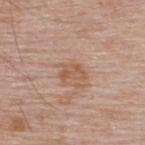The lesion was photographed on a routine skin check and not biopsied; there is no pathology result. Located on the upper back. A 15 mm close-up tile from a total-body photography series done for melanoma screening. A male patient, about 65 years old. About 3 mm across.